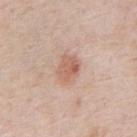No biopsy was performed on this lesion — it was imaged during a full skin examination and was not determined to be concerning. Located on the chest. This is a white-light tile. A male subject, approximately 80 years of age. Cropped from a whole-body photographic skin survey; the tile spans about 15 mm.